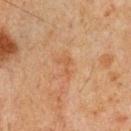| key | value |
|---|---|
| biopsy status | imaged on a skin check; not biopsied |
| diameter | ≈2.5 mm |
| image source | 15 mm crop, total-body photography |
| automated metrics | border irregularity of about 6.5 on a 0–10 scale and a color-variation rating of about 0/10; an automated nevus-likeness rating near 0 out of 100 |
| anatomic site | the chest |
| subject | male, in their mid- to late 60s |